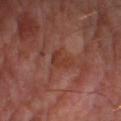{"biopsy_status": "not biopsied; imaged during a skin examination", "image": {"source": "total-body photography crop", "field_of_view_mm": 15}, "lesion_size": {"long_diameter_mm_approx": 3.0}, "site": "right lower leg", "patient": {"sex": "male", "age_approx": 70}}